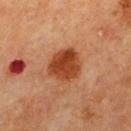Impression: The lesion was tiled from a total-body skin photograph and was not biopsied. Context: Imaged with cross-polarized lighting. The lesion is on the mid back. A male subject roughly 65 years of age. Cropped from a whole-body photographic skin survey; the tile spans about 15 mm.